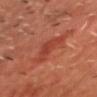Recorded during total-body skin imaging; not selected for excision or biopsy. A male patient aged 43 to 47. This image is a 15 mm lesion crop taken from a total-body photograph. The lesion is located on the chest.